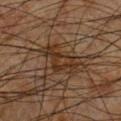Findings:
– workup: catalogued during a skin exam; not biopsied
– anatomic site: the front of the torso
– illumination: cross-polarized illumination
– subject: male, aged 48–52
– size: ≈4.5 mm
– image source: 15 mm crop, total-body photography